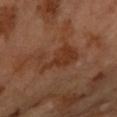Imaged during a routine full-body skin examination; the lesion was not biopsied and no histopathology is available. On the arm. Imaged with cross-polarized lighting. The recorded lesion diameter is about 5.5 mm. A female patient aged 58 to 62. Automated image analysis of the tile measured a lesion area of about 12 mm², an eccentricity of roughly 0.85, and a symmetry-axis asymmetry near 0.45. It also reported a lesion color around L≈36 a*≈22 b*≈32 in CIELAB and a lesion-to-skin contrast of about 7 (normalized; higher = more distinct). It also reported a border-irregularity rating of about 7/10 and a within-lesion color-variation index near 2.5/10. The analysis additionally found an automated nevus-likeness rating near 0 out of 100 and a lesion-detection confidence of about 100/100. Cropped from a total-body skin-imaging series; the visible field is about 15 mm.